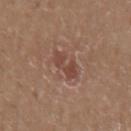Captured under white-light illumination. A 15 mm crop from a total-body photograph taken for skin-cancer surveillance. The lesion is located on the mid back. The recorded lesion diameter is about 3.5 mm. A male subject aged around 20.